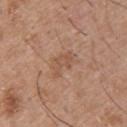The lesion was photographed on a routine skin check and not biopsied; there is no pathology result.